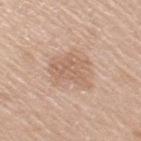<tbp_lesion>
<biopsy_status>not biopsied; imaged during a skin examination</biopsy_status>
<image>
  <source>total-body photography crop</source>
  <field_of_view_mm>15</field_of_view_mm>
</image>
<patient>
  <sex>male</sex>
  <age_approx>60</age_approx>
</patient>
<automated_metrics>
  <area_mm2_approx>13.0</area_mm2_approx>
  <eccentricity>0.75</eccentricity>
  <shape_asymmetry>0.35</shape_asymmetry>
  <lesion_detection_confidence_0_100>100</lesion_detection_confidence_0_100>
</automated_metrics>
<lighting>white-light</lighting>
<site>arm</site>
</tbp_lesion>